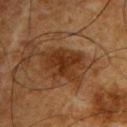{"biopsy_status": "not biopsied; imaged during a skin examination", "image": {"source": "total-body photography crop", "field_of_view_mm": 15}, "patient": {"sex": "male", "age_approx": 65}, "automated_metrics": {"cielab_L": 26, "cielab_a": 18, "cielab_b": 28, "vs_skin_darker_L": 8.0, "vs_skin_contrast_norm": 9.0, "nevus_likeness_0_100": 0}, "lesion_size": {"long_diameter_mm_approx": 4.5}, "site": "right upper arm", "lighting": "cross-polarized"}